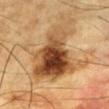Findings:
– follow-up: catalogued during a skin exam; not biopsied
– subject: male, about 85 years old or older
– image: ~15 mm tile from a whole-body skin photo
– anatomic site: the chest